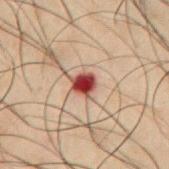  lesion_size:
    long_diameter_mm_approx: 2.5
  patient:
    sex: male
    age_approx: 50
  image:
    source: total-body photography crop
    field_of_view_mm: 15
  site: abdomen
  lighting: cross-polarized